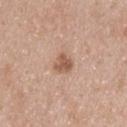Imaged during a routine full-body skin examination; the lesion was not biopsied and no histopathology is available. A male subject, aged approximately 45. The lesion is on the upper back. Automated tile analysis of the lesion measured a classifier nevus-likeness of about 60/100 and a detector confidence of about 100 out of 100 that the crop contains a lesion. This is a white-light tile. A region of skin cropped from a whole-body photographic capture, roughly 15 mm wide.